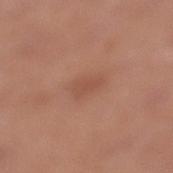follow-up: catalogued during a skin exam; not biopsied | illumination: white-light illumination | lesion size: ≈2.5 mm | patient: female, aged approximately 40 | image-analysis metrics: a footprint of about 4 mm², an outline eccentricity of about 0.8 (0 = round, 1 = elongated), and two-axis asymmetry of about 0.3; a border-irregularity rating of about 3/10, a color-variation rating of about 1/10, and radial color variation of about 0.5; a classifier nevus-likeness of about 5/100 and a lesion-detection confidence of about 100/100 | location: the left lower leg | acquisition: ~15 mm crop, total-body skin-cancer survey.